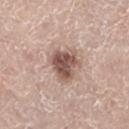Captured during whole-body skin photography for melanoma surveillance; the lesion was not biopsied. About 4.5 mm across. The lesion is on the right leg. This image is a 15 mm lesion crop taken from a total-body photograph. A male subject aged 68–72.